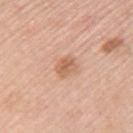biopsy_status: not biopsied; imaged during a skin examination
site: left upper arm
image:
  source: total-body photography crop
  field_of_view_mm: 15
patient:
  sex: female
  age_approx: 65
automated_metrics:
  area_mm2_approx: 4.5
  eccentricity: 0.6
  cielab_L: 62
  cielab_a: 22
  cielab_b: 34
  vs_skin_darker_L: 10.0
  vs_skin_contrast_norm: 7.0
  border_irregularity_0_10: 2.0
  color_variation_0_10: 4.0
  peripheral_color_asymmetry: 1.5